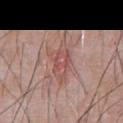  biopsy_status: not biopsied; imaged during a skin examination
  automated_metrics:
    area_mm2_approx: 11.0
    eccentricity: 0.8
    shape_asymmetry: 0.35
    border_irregularity_0_10: 4.5
    color_variation_0_10: 4.0
    peripheral_color_asymmetry: 1.0
  site: abdomen
  patient:
    sex: male
    age_approx: 60
  image:
    source: total-body photography crop
    field_of_view_mm: 15
  lesion_size:
    long_diameter_mm_approx: 5.0